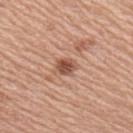Recorded during total-body skin imaging; not selected for excision or biopsy. Automated image analysis of the tile measured an area of roughly 4 mm², an outline eccentricity of about 0.65 (0 = round, 1 = elongated), and a symmetry-axis asymmetry near 0.25. It also reported a lesion color around L≈52 a*≈23 b*≈30 in CIELAB, a lesion–skin lightness drop of about 13, and a normalized border contrast of about 9. It also reported a border-irregularity index near 2/10, a within-lesion color-variation index near 4/10, and a peripheral color-asymmetry measure near 1.5. And it measured lesion-presence confidence of about 100/100. This image is a 15 mm lesion crop taken from a total-body photograph. The patient is a female roughly 65 years of age. Captured under white-light illumination. The lesion is on the right upper arm. Measured at roughly 2.5 mm in maximum diameter.